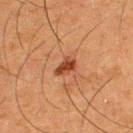<lesion>
<biopsy_status>not biopsied; imaged during a skin examination</biopsy_status>
<lighting>cross-polarized</lighting>
<lesion_size>
  <long_diameter_mm_approx>3.0</long_diameter_mm_approx>
</lesion_size>
<image>
  <source>total-body photography crop</source>
  <field_of_view_mm>15</field_of_view_mm>
</image>
<patient>
  <sex>male</sex>
  <age_approx>35</age_approx>
</patient>
<site>back</site>
</lesion>